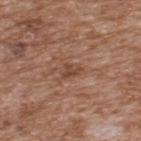{
  "biopsy_status": "not biopsied; imaged during a skin examination",
  "automated_metrics": {
    "area_mm2_approx": 4.0,
    "eccentricity": 0.7,
    "lesion_detection_confidence_0_100": 100
  },
  "image": {
    "source": "total-body photography crop",
    "field_of_view_mm": 15
  },
  "site": "back",
  "patient": {
    "sex": "male",
    "age_approx": 65
  },
  "lighting": "white-light",
  "lesion_size": {
    "long_diameter_mm_approx": 2.5
  }
}